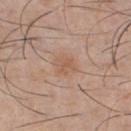biopsy status: catalogued during a skin exam; not biopsied | site: the chest | subject: male, approximately 45 years of age | illumination: white-light | automated metrics: a footprint of about 4 mm², a shape eccentricity near 0.55, and a shape-asymmetry score of about 0.25 (0 = symmetric); a border-irregularity index near 2.5/10 | lesion diameter: ≈2.5 mm | acquisition: 15 mm crop, total-body photography.